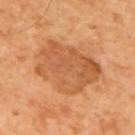The lesion was photographed on a routine skin check and not biopsied; there is no pathology result. A male subject, aged 63–67. Located on the upper back. Cropped from a whole-body photographic skin survey; the tile spans about 15 mm.